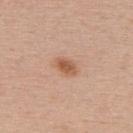Clinical impression: Imaged during a routine full-body skin examination; the lesion was not biopsied and no histopathology is available. Acquisition and patient details: On the upper back. Cropped from a whole-body photographic skin survey; the tile spans about 15 mm. Automated image analysis of the tile measured an area of roughly 5 mm². The analysis additionally found a border-irregularity index near 2/10, internal color variation of about 3 on a 0–10 scale, and radial color variation of about 1. The tile uses white-light illumination. The lesion's longest dimension is about 3 mm. The patient is a female aged 38–42.